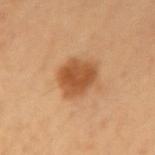This lesion was catalogued during total-body skin photography and was not selected for biopsy.
A roughly 15 mm field-of-view crop from a total-body skin photograph.
On the left forearm.
The subject is a female aged 38–42.